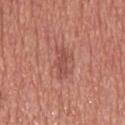notes = catalogued during a skin exam; not biopsied
imaging modality = total-body-photography crop, ~15 mm field of view
patient = male, aged 48–52
location = the head or neck